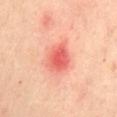Assessment:
This lesion was catalogued during total-body skin photography and was not selected for biopsy.
Acquisition and patient details:
A close-up tile cropped from a whole-body skin photograph, about 15 mm across. The lesion is located on the back. A female subject, in their 40s.